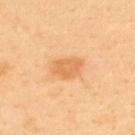Impression:
The lesion was photographed on a routine skin check and not biopsied; there is no pathology result.
Clinical summary:
The tile uses cross-polarized illumination. About 3.5 mm across. A male patient in their 40s. Cropped from a whole-body photographic skin survey; the tile spans about 15 mm. The lesion is on the back.